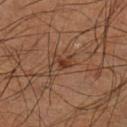The lesion was tiled from a total-body skin photograph and was not biopsied. A male subject, aged 63 to 67. A 15 mm close-up tile from a total-body photography series done for melanoma screening. The lesion is located on the left lower leg.